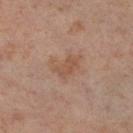Part of a total-body skin-imaging series; this lesion was reviewed on a skin check and was not flagged for biopsy.
A female patient roughly 55 years of age.
A 15 mm close-up tile from a total-body photography series done for melanoma screening.
The lesion-visualizer software estimated an area of roughly 7 mm², an eccentricity of roughly 0.75, and two-axis asymmetry of about 0.4. And it measured an automated nevus-likeness rating near 0 out of 100.
On the right thigh.
Captured under cross-polarized illumination.
The lesion's longest dimension is about 4 mm.